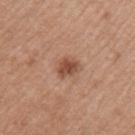Findings:
- follow-up: catalogued during a skin exam; not biopsied
- diameter: about 3 mm
- acquisition: total-body-photography crop, ~15 mm field of view
- patient: female, aged 48 to 52
- tile lighting: white-light illumination
- image-analysis metrics: an eccentricity of roughly 0.65 and a symmetry-axis asymmetry near 0.25; a peripheral color-asymmetry measure near 1; an automated nevus-likeness rating near 85 out of 100
- anatomic site: the left upper arm